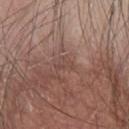biopsy_status: not biopsied; imaged during a skin examination
patient:
  sex: male
  age_approx: 45
image:
  source: total-body photography crop
  field_of_view_mm: 15
site: chest